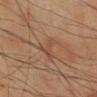patient:
  sex: male
  age_approx: 70
lesion_size:
  long_diameter_mm_approx: 3.5
site: right lower leg
automated_metrics:
  cielab_L: 44
  cielab_a: 19
  cielab_b: 29
  vs_skin_contrast_norm: 5.0
  color_variation_0_10: 1.0
  peripheral_color_asymmetry: 0.0
  lesion_detection_confidence_0_100: 85
image:
  source: total-body photography crop
  field_of_view_mm: 15
lighting: cross-polarized